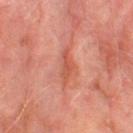The lesion was photographed on a routine skin check and not biopsied; there is no pathology result. A region of skin cropped from a whole-body photographic capture, roughly 15 mm wide. Captured under cross-polarized illumination. The lesion's longest dimension is about 3.5 mm. On the right thigh. A male subject aged around 75. Automated tile analysis of the lesion measured a lesion area of about 4 mm², a shape eccentricity near 0.9, and two-axis asymmetry of about 0.4.